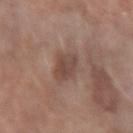Clinical impression: Captured during whole-body skin photography for melanoma surveillance; the lesion was not biopsied. Clinical summary: The tile uses white-light illumination. Located on the left forearm. A female patient about 60 years old. A region of skin cropped from a whole-body photographic capture, roughly 15 mm wide.